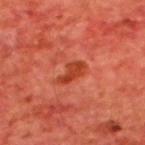Part of a total-body skin-imaging series; this lesion was reviewed on a skin check and was not flagged for biopsy.
Imaged with cross-polarized lighting.
The recorded lesion diameter is about 3.5 mm.
The subject is a male aged 63–67.
A 15 mm close-up extracted from a 3D total-body photography capture.
Located on the upper back.